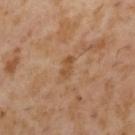No biopsy was performed on this lesion — it was imaged during a full skin examination and was not determined to be concerning.
Located on the arm.
A 15 mm close-up extracted from a 3D total-body photography capture.
This is a cross-polarized tile.
The patient is a male about 55 years old.
Measured at roughly 3 mm in maximum diameter.